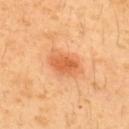Imaged during a routine full-body skin examination; the lesion was not biopsied and no histopathology is available.
A male patient, in their 30s.
The recorded lesion diameter is about 3.5 mm.
The lesion is on the upper back.
A 15 mm close-up tile from a total-body photography series done for melanoma screening.
An algorithmic analysis of the crop reported a mean CIELAB color near L≈60 a*≈31 b*≈43, roughly 11 lightness units darker than nearby skin, and a normalized lesion–skin contrast near 7. It also reported a border-irregularity rating of about 2.5/10 and internal color variation of about 3 on a 0–10 scale. The software also gave a classifier nevus-likeness of about 100/100.